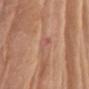Findings:
- body site: the left upper arm
- image: total-body-photography crop, ~15 mm field of view
- subject: female, roughly 80 years of age
- size: ≈2.5 mm
- lighting: white-light illumination
- image-analysis metrics: an average lesion color of about L≈56 a*≈23 b*≈28 (CIELAB) and about 6 CIELAB-L* units darker than the surrounding skin; a border-irregularity index near 4/10, a within-lesion color-variation index near 3.5/10, and peripheral color asymmetry of about 1; a classifier nevus-likeness of about 0/100 and a detector confidence of about 95 out of 100 that the crop contains a lesion